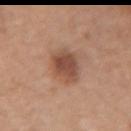Background:
A region of skin cropped from a whole-body photographic capture, roughly 15 mm wide. The patient is a female aged around 60. Automated image analysis of the tile measured a classifier nevus-likeness of about 85/100 and a lesion-detection confidence of about 100/100. From the left forearm.